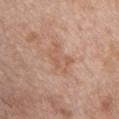notes: total-body-photography surveillance lesion; no biopsy | illumination: white-light | size: about 4 mm | subject: female, aged around 40 | image-analysis metrics: a lesion area of about 6 mm², a shape eccentricity near 0.8, and a shape-asymmetry score of about 0.5 (0 = symmetric); an average lesion color of about L≈58 a*≈21 b*≈30 (CIELAB), a lesion–skin lightness drop of about 7, and a normalized lesion–skin contrast near 5; border irregularity of about 7 on a 0–10 scale, internal color variation of about 3 on a 0–10 scale, and radial color variation of about 1 | imaging modality: ~15 mm crop, total-body skin-cancer survey | site: the chest.